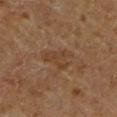Imaged during a routine full-body skin examination; the lesion was not biopsied and no histopathology is available. An algorithmic analysis of the crop reported an area of roughly 7.5 mm², an outline eccentricity of about 0.8 (0 = round, 1 = elongated), and a shape-asymmetry score of about 0.45 (0 = symmetric). It also reported a lesion color around L≈36 a*≈16 b*≈28 in CIELAB and a lesion-to-skin contrast of about 5 (normalized; higher = more distinct). Imaged with cross-polarized lighting. The lesion is on the chest. About 4 mm across. A 15 mm close-up tile from a total-body photography series done for melanoma screening. A male subject aged approximately 65.